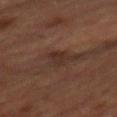This lesion was catalogued during total-body skin photography and was not selected for biopsy. Cropped from a whole-body photographic skin survey; the tile spans about 15 mm. An algorithmic analysis of the crop reported a shape eccentricity near 0.8 and two-axis asymmetry of about 0.3. The software also gave a color-variation rating of about 2/10. And it measured a nevus-likeness score of about 5/100 and lesion-presence confidence of about 95/100. Captured under cross-polarized illumination. Approximately 2.5 mm at its widest. A male subject aged 63–67. From the back.